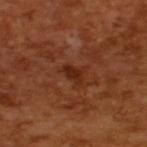The lesion was photographed on a routine skin check and not biopsied; there is no pathology result. A male subject in their mid-60s. The recorded lesion diameter is about 2.5 mm. The tile uses cross-polarized illumination. A 15 mm close-up extracted from a 3D total-body photography capture.